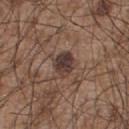No biopsy was performed on this lesion — it was imaged during a full skin examination and was not determined to be concerning.
The subject is a male roughly 55 years of age.
Located on the chest.
A roughly 15 mm field-of-view crop from a total-body skin photograph.